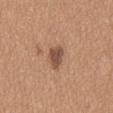- notes: imaged on a skin check; not biopsied
- lesion diameter: ~3.5 mm (longest diameter)
- site: the mid back
- patient: female, roughly 45 years of age
- image: ~15 mm crop, total-body skin-cancer survey